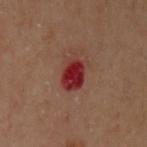notes = no biopsy performed (imaged during a skin exam) | patient = female, aged around 60 | TBP lesion metrics = about 11 CIELAB-L* units darker than the surrounding skin; internal color variation of about 4.5 on a 0–10 scale and radial color variation of about 1.5; a classifier nevus-likeness of about 0/100 and a lesion-detection confidence of about 100/100 | site = the right arm | diameter = about 3.5 mm | imaging modality = total-body-photography crop, ~15 mm field of view | lighting = cross-polarized.